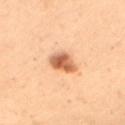{"biopsy_status": "not biopsied; imaged during a skin examination", "image": {"source": "total-body photography crop", "field_of_view_mm": 15}, "patient": {"sex": "female", "age_approx": 50}, "site": "mid back"}